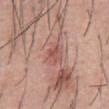This lesion was catalogued during total-body skin photography and was not selected for biopsy.
A 15 mm crop from a total-body photograph taken for skin-cancer surveillance.
A male patient aged approximately 55.
From the front of the torso.